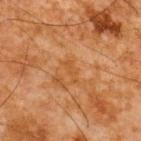Clinical impression: Captured during whole-body skin photography for melanoma surveillance; the lesion was not biopsied. Image and clinical context: The subject is a male aged around 65. This image is a 15 mm lesion crop taken from a total-body photograph. Located on the back.